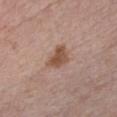Cropped from a total-body skin-imaging series; the visible field is about 15 mm. The patient is a male aged 58–62. Located on the front of the torso. This is a white-light tile. About 3 mm across.